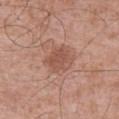Q: Was this lesion biopsied?
A: total-body-photography surveillance lesion; no biopsy
Q: What kind of image is this?
A: 15 mm crop, total-body photography
Q: What did automated image analysis measure?
A: a footprint of about 10 mm² and a symmetry-axis asymmetry near 0.2; a mean CIELAB color near L≈53 a*≈22 b*≈28, roughly 8 lightness units darker than nearby skin, and a normalized border contrast of about 6; a classifier nevus-likeness of about 20/100 and a detector confidence of about 100 out of 100 that the crop contains a lesion
Q: Who is the patient?
A: male, aged approximately 70
Q: Where on the body is the lesion?
A: the abdomen
Q: How large is the lesion?
A: ≈3.5 mm
Q: How was the tile lit?
A: white-light illumination